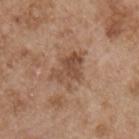Case summary:
* notes · imaged on a skin check; not biopsied
* imaging modality · 15 mm crop, total-body photography
* patient · male, about 55 years old
* location · the chest